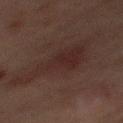No biopsy was performed on this lesion — it was imaged during a full skin examination and was not determined to be concerning.